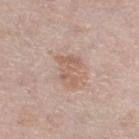This lesion was catalogued during total-body skin photography and was not selected for biopsy.
A roughly 15 mm field-of-view crop from a total-body skin photograph.
A female subject aged 63–67.
Imaged with white-light lighting.
The lesion is located on the leg.
Approximately 4 mm at its widest.